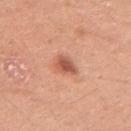<record>
  <biopsy_status>not biopsied; imaged during a skin examination</biopsy_status>
  <site>left upper arm</site>
  <image>
    <source>total-body photography crop</source>
    <field_of_view_mm>15</field_of_view_mm>
  </image>
  <patient>
    <sex>female</sex>
    <age_approx>40</age_approx>
  </patient>
  <lighting>white-light</lighting>
  <lesion_size>
    <long_diameter_mm_approx>3.0</long_diameter_mm_approx>
  </lesion_size>
</record>